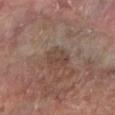follow-up = catalogued during a skin exam; not biopsied
patient = female, aged 83 or older
image = 15 mm crop, total-body photography
diameter = ≈2.5 mm
tile lighting = white-light
body site = the right forearm